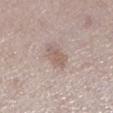Assessment:
No biopsy was performed on this lesion — it was imaged during a full skin examination and was not determined to be concerning.
Context:
The tile uses white-light illumination. The patient is a female aged around 50. Automated tile analysis of the lesion measured an area of roughly 4 mm², a shape eccentricity near 0.85, and two-axis asymmetry of about 0.25. The analysis additionally found an automated nevus-likeness rating near 30 out of 100 and lesion-presence confidence of about 95/100. Longest diameter approximately 3 mm. Cropped from a total-body skin-imaging series; the visible field is about 15 mm. On the left lower leg.